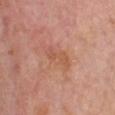biopsy status: total-body-photography surveillance lesion; no biopsy | automated lesion analysis: a lesion area of about 5 mm², a shape eccentricity near 0.9, and a symmetry-axis asymmetry near 0.25; a lesion-to-skin contrast of about 5 (normalized; higher = more distinct); a border-irregularity rating of about 3/10, internal color variation of about 3 on a 0–10 scale, and peripheral color asymmetry of about 0.5; a nevus-likeness score of about 0/100 | tile lighting: white-light illumination | imaging modality: ~15 mm tile from a whole-body skin photo | anatomic site: the chest | patient: male, about 75 years old | lesion size: ~3.5 mm (longest diameter).